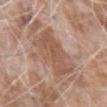Q: Is there a histopathology result?
A: catalogued during a skin exam; not biopsied
Q: How was this image acquired?
A: ~15 mm tile from a whole-body skin photo
Q: What are the patient's age and sex?
A: male, aged 73–77
Q: What is the lesion's diameter?
A: about 5.5 mm
Q: Lesion location?
A: the left forearm
Q: What did automated image analysis measure?
A: a lesion area of about 21 mm², an eccentricity of roughly 0.6, and a shape-asymmetry score of about 0.25 (0 = symmetric); a mean CIELAB color near L≈54 a*≈19 b*≈29, roughly 8 lightness units darker than nearby skin, and a normalized lesion–skin contrast near 6; a detector confidence of about 85 out of 100 that the crop contains a lesion